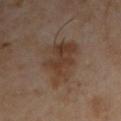Impression: Recorded during total-body skin imaging; not selected for excision or biopsy. Acquisition and patient details: The total-body-photography lesion software estimated a mean CIELAB color near L≈38 a*≈15 b*≈26, about 8 CIELAB-L* units darker than the surrounding skin, and a lesion-to-skin contrast of about 7.5 (normalized; higher = more distinct). About 6.5 mm across. A region of skin cropped from a whole-body photographic capture, roughly 15 mm wide. The subject is a male aged 53–57. This is a cross-polarized tile. From the arm.